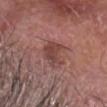| feature | finding |
|---|---|
| notes | total-body-photography surveillance lesion; no biopsy |